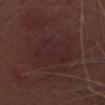On excision, pathology confirmed a seborrheic keratosis.